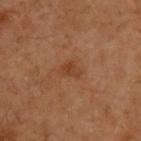Part of a total-body skin-imaging series; this lesion was reviewed on a skin check and was not flagged for biopsy.
A male patient, about 60 years old.
A 15 mm crop from a total-body photograph taken for skin-cancer surveillance.
Imaged with cross-polarized lighting.
From the upper back.
The total-body-photography lesion software estimated a lesion area of about 4 mm² and a shape eccentricity near 0.75. It also reported a lesion-to-skin contrast of about 6 (normalized; higher = more distinct). And it measured a border-irregularity index near 3.5/10, a within-lesion color-variation index near 2.5/10, and radial color variation of about 1. It also reported an automated nevus-likeness rating near 5 out of 100.